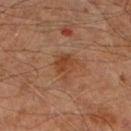follow-up: no biopsy performed (imaged during a skin exam) | subject: male, aged around 65 | automated metrics: a footprint of about 6.5 mm², an eccentricity of roughly 0.55, and two-axis asymmetry of about 0.35; border irregularity of about 3.5 on a 0–10 scale and a color-variation rating of about 3/10; a nevus-likeness score of about 40/100 and a detector confidence of about 100 out of 100 that the crop contains a lesion | imaging modality: total-body-photography crop, ~15 mm field of view | lighting: cross-polarized | lesion diameter: ≈3.5 mm | site: the right lower leg.